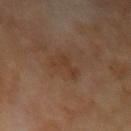Notes:
* follow-up · no biopsy performed (imaged during a skin exam)
* image · total-body-photography crop, ~15 mm field of view
* lesion size · ~3 mm (longest diameter)
* subject · male, aged 68 to 72
* anatomic site · the left upper arm
* illumination · cross-polarized illumination